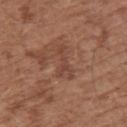biopsy status = imaged on a skin check; not biopsied | imaging modality = ~15 mm tile from a whole-body skin photo | location = the upper back | patient = male, aged 73 to 77.